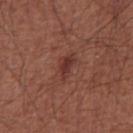The lesion was photographed on a routine skin check and not biopsied; there is no pathology result.
From the front of the torso.
Cropped from a whole-body photographic skin survey; the tile spans about 15 mm.
The subject is a male aged 63–67.
An algorithmic analysis of the crop reported a mean CIELAB color near L≈35 a*≈24 b*≈24 and a normalized border contrast of about 7.5.
This is a white-light tile.
Measured at roughly 3 mm in maximum diameter.